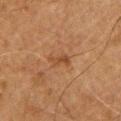Part of a total-body skin-imaging series; this lesion was reviewed on a skin check and was not flagged for biopsy.
From the chest.
A male patient, aged around 65.
Longest diameter approximately 3 mm.
A region of skin cropped from a whole-body photographic capture, roughly 15 mm wide.
Automated tile analysis of the lesion measured a within-lesion color-variation index near 1.5/10 and radial color variation of about 0.5.